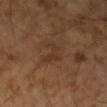Recorded during total-body skin imaging; not selected for excision or biopsy. An algorithmic analysis of the crop reported a footprint of about 4 mm², an outline eccentricity of about 0.8 (0 = round, 1 = elongated), and a symmetry-axis asymmetry near 0.5. The software also gave an average lesion color of about L≈32 a*≈18 b*≈28 (CIELAB), about 5 CIELAB-L* units darker than the surrounding skin, and a normalized border contrast of about 5. Cropped from a total-body skin-imaging series; the visible field is about 15 mm. Measured at roughly 3 mm in maximum diameter. A male subject, approximately 60 years of age. The lesion is on the arm.